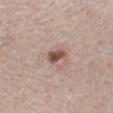follow-up=catalogued during a skin exam; not biopsied | automated lesion analysis=a color-variation rating of about 4/10 and a peripheral color-asymmetry measure near 1.5 | tile lighting=white-light illumination | imaging modality=15 mm crop, total-body photography | patient=female, aged 28–32 | lesion size=≈3 mm | site=the mid back.